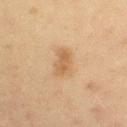Impression:
Recorded during total-body skin imaging; not selected for excision or biopsy.
Background:
On the left upper arm. A lesion tile, about 15 mm wide, cut from a 3D total-body photograph. The recorded lesion diameter is about 4 mm. A female patient, aged 18 to 22. Imaged with cross-polarized lighting.